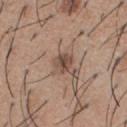Imaged during a routine full-body skin examination; the lesion was not biopsied and no histopathology is available.
The lesion's longest dimension is about 2.5 mm.
The total-body-photography lesion software estimated a classifier nevus-likeness of about 50/100 and a lesion-detection confidence of about 100/100.
On the chest.
A male subject aged 58–62.
A 15 mm close-up extracted from a 3D total-body photography capture.